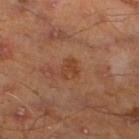| feature | finding |
|---|---|
| workup | catalogued during a skin exam; not biopsied |
| imaging modality | ~15 mm tile from a whole-body skin photo |
| site | the left lower leg |
| TBP lesion metrics | a lesion area of about 3.5 mm² and two-axis asymmetry of about 0.35; a classifier nevus-likeness of about 10/100 and a detector confidence of about 100 out of 100 that the crop contains a lesion |
| patient | male, in their mid- to late 40s |
| lighting | cross-polarized |